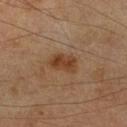{"image": {"source": "total-body photography crop", "field_of_view_mm": 15}, "patient": {"sex": "male", "age_approx": 65}, "lighting": "cross-polarized", "site": "right lower leg", "automated_metrics": {"cielab_L": 39, "cielab_a": 19, "cielab_b": 32, "vs_skin_darker_L": 9.0, "vs_skin_contrast_norm": 8.0, "nevus_likeness_0_100": 80, "lesion_detection_confidence_0_100": 100}, "lesion_size": {"long_diameter_mm_approx": 3.5}}